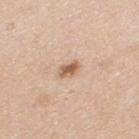Impression:
The lesion was tiled from a total-body skin photograph and was not biopsied.
Background:
A male patient, aged 33–37. This is a white-light tile. Located on the upper back. A 15 mm close-up extracted from a 3D total-body photography capture. The recorded lesion diameter is about 2.5 mm.